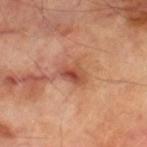Assessment:
Part of a total-body skin-imaging series; this lesion was reviewed on a skin check and was not flagged for biopsy.
Acquisition and patient details:
A male patient approximately 70 years of age. The lesion is located on the leg. A 15 mm crop from a total-body photograph taken for skin-cancer surveillance. The tile uses cross-polarized illumination.